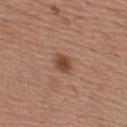A male patient approximately 55 years of age.
Automated tile analysis of the lesion measured a footprint of about 4.5 mm², an outline eccentricity of about 0.55 (0 = round, 1 = elongated), and two-axis asymmetry of about 0.25.
Approximately 2.5 mm at its widest.
A close-up tile cropped from a whole-body skin photograph, about 15 mm across.
From the upper back.